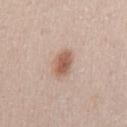{"biopsy_status": "not biopsied; imaged during a skin examination", "image": {"source": "total-body photography crop", "field_of_view_mm": 15}, "patient": {"sex": "female", "age_approx": 30}, "site": "mid back"}